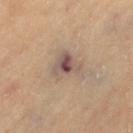follow-up: catalogued during a skin exam; not biopsied | location: the left thigh | subject: female, about 70 years old | lighting: cross-polarized illumination | automated metrics: a border-irregularity rating of about 4/10, a color-variation rating of about 9/10, and a peripheral color-asymmetry measure near 2.5; a nevus-likeness score of about 10/100 and a lesion-detection confidence of about 100/100 | lesion diameter: ≈4 mm | imaging modality: total-body-photography crop, ~15 mm field of view.